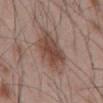Assessment: No biopsy was performed on this lesion — it was imaged during a full skin examination and was not determined to be concerning. Context: From the front of the torso. A male patient, aged around 55. A roughly 15 mm field-of-view crop from a total-body skin photograph.